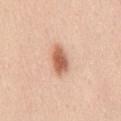biopsy status: catalogued during a skin exam; not biopsied | location: the mid back | lesion diameter: ≈4 mm | patient: female, about 40 years old | automated lesion analysis: an area of roughly 6.5 mm², a shape eccentricity near 0.85, and two-axis asymmetry of about 0.25; lesion-presence confidence of about 100/100 | lighting: white-light illumination | acquisition: total-body-photography crop, ~15 mm field of view.